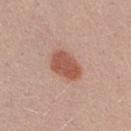The lesion was photographed on a routine skin check and not biopsied; there is no pathology result.
Cropped from a total-body skin-imaging series; the visible field is about 15 mm.
The lesion is located on the right thigh.
An algorithmic analysis of the crop reported a lesion color around L≈54 a*≈25 b*≈29 in CIELAB, roughly 12 lightness units darker than nearby skin, and a lesion-to-skin contrast of about 8.5 (normalized; higher = more distinct). The analysis additionally found border irregularity of about 1.5 on a 0–10 scale, a color-variation rating of about 2.5/10, and radial color variation of about 1. The analysis additionally found an automated nevus-likeness rating near 100 out of 100.
A female patient about 25 years old.
Imaged with white-light lighting.
The recorded lesion diameter is about 4 mm.